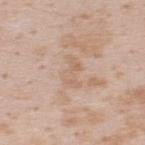Part of a total-body skin-imaging series; this lesion was reviewed on a skin check and was not flagged for biopsy.
The tile uses white-light illumination.
Longest diameter approximately 3.5 mm.
The subject is a male aged approximately 35.
A lesion tile, about 15 mm wide, cut from a 3D total-body photograph.
On the upper back.